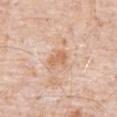– biopsy status — total-body-photography surveillance lesion; no biopsy
– body site — the abdomen
– image — 15 mm crop, total-body photography
– subject — male, approximately 80 years of age